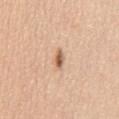follow-up = catalogued during a skin exam; not biopsied
diameter = ≈2.5 mm
automated lesion analysis = a lesion area of about 3 mm², a shape eccentricity near 0.9, and a symmetry-axis asymmetry near 0.35; an average lesion color of about L≈61 a*≈21 b*≈33 (CIELAB), about 14 CIELAB-L* units darker than the surrounding skin, and a normalized lesion–skin contrast near 8.5; a border-irregularity index near 3.5/10, a within-lesion color-variation index near 3/10, and peripheral color asymmetry of about 1; a nevus-likeness score of about 95/100 and lesion-presence confidence of about 100/100
subject = male, approximately 60 years of age
image source = ~15 mm crop, total-body skin-cancer survey
anatomic site = the abdomen